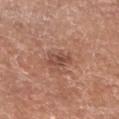| feature | finding |
|---|---|
| workup | catalogued during a skin exam; not biopsied |
| image source | ~15 mm crop, total-body skin-cancer survey |
| subject | male, aged approximately 70 |
| automated lesion analysis | an average lesion color of about L≈48 a*≈23 b*≈27 (CIELAB), a lesion–skin lightness drop of about 10, and a lesion-to-skin contrast of about 7 (normalized; higher = more distinct); a classifier nevus-likeness of about 5/100 |
| diameter | ≈3.5 mm |
| illumination | white-light illumination |
| anatomic site | the leg |